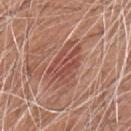Impression:
This lesion was catalogued during total-body skin photography and was not selected for biopsy.
Acquisition and patient details:
The lesion is located on the front of the torso. Captured under white-light illumination. The subject is a male approximately 60 years of age. Automated image analysis of the tile measured an area of roughly 13 mm², an eccentricity of roughly 0.8, and two-axis asymmetry of about 0.3. The software also gave a border-irregularity index near 6.5/10, a color-variation rating of about 4/10, and peripheral color asymmetry of about 1.5. The analysis additionally found an automated nevus-likeness rating near 15 out of 100 and a lesion-detection confidence of about 100/100. The lesion's longest dimension is about 5.5 mm. A close-up tile cropped from a whole-body skin photograph, about 15 mm across.